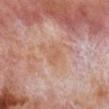Clinical impression:
This lesion was catalogued during total-body skin photography and was not selected for biopsy.
Clinical summary:
The lesion is on the right lower leg. About 3 mm across. A close-up tile cropped from a whole-body skin photograph, about 15 mm across. The tile uses cross-polarized illumination. A male patient about 70 years old.